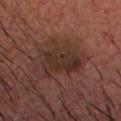Assessment:
Recorded during total-body skin imaging; not selected for excision or biopsy.
Image and clinical context:
Automated image analysis of the tile measured a lesion area of about 20 mm² and an outline eccentricity of about 0.6 (0 = round, 1 = elongated). The tile uses cross-polarized illumination. A male subject, aged 43 to 47. Located on the head or neck. The recorded lesion diameter is about 6 mm. A 15 mm close-up tile from a total-body photography series done for melanoma screening.